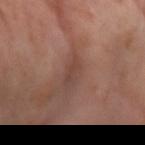Located on the arm. The recorded lesion diameter is about 3 mm. A roughly 15 mm field-of-view crop from a total-body skin photograph. A female patient, approximately 55 years of age.